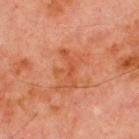<lesion>
<biopsy_status>not biopsied; imaged during a skin examination</biopsy_status>
<lighting>cross-polarized</lighting>
<site>chest</site>
<image>
  <source>total-body photography crop</source>
  <field_of_view_mm>15</field_of_view_mm>
</image>
<patient>
  <sex>male</sex>
  <age_approx>70</age_approx>
</patient>
<automated_metrics>
  <area_mm2_approx>5.0</area_mm2_approx>
  <eccentricity>0.95</eccentricity>
  <shape_asymmetry>0.6</shape_asymmetry>
</automated_metrics>
</lesion>